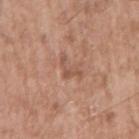Located on the right upper arm. The tile uses white-light illumination. Automated image analysis of the tile measured a border-irregularity index near 8.5/10, a color-variation rating of about 0/10, and peripheral color asymmetry of about 0. And it measured a nevus-likeness score of about 0/100 and lesion-presence confidence of about 100/100. The recorded lesion diameter is about 3 mm. A male subject, aged approximately 55. Cropped from a total-body skin-imaging series; the visible field is about 15 mm.